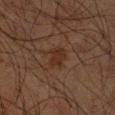subject — male, in their mid-60s; acquisition — ~15 mm tile from a whole-body skin photo; body site — the right upper arm.